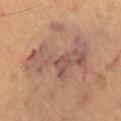Q: Is there a histopathology result?
A: total-body-photography surveillance lesion; no biopsy
Q: How was this image acquired?
A: ~15 mm tile from a whole-body skin photo
Q: Patient demographics?
A: male, in their 60s
Q: Lesion location?
A: the leg
Q: Automated lesion metrics?
A: a lesion area of about 22 mm², an eccentricity of roughly 0.9, and a shape-asymmetry score of about 0.5 (0 = symmetric); a mean CIELAB color near L≈51 a*≈19 b*≈25, roughly 9 lightness units darker than nearby skin, and a normalized lesion–skin contrast near 7.5